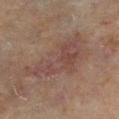Notes:
* biopsy status · no biopsy performed (imaged during a skin exam)
* site · the leg
* size · ~9 mm (longest diameter)
* illumination · cross-polarized illumination
* imaging modality · total-body-photography crop, ~15 mm field of view
* patient · female, aged approximately 60